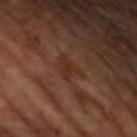Impression: The lesion was photographed on a routine skin check and not biopsied; there is no pathology result. Clinical summary: The patient is a male in their mid- to late 50s. The total-body-photography lesion software estimated an area of roughly 6 mm², an outline eccentricity of about 0.7 (0 = round, 1 = elongated), and a shape-asymmetry score of about 0.45 (0 = symmetric). And it measured a lesion color around L≈30 a*≈19 b*≈26 in CIELAB, roughly 5 lightness units darker than nearby skin, and a normalized border contrast of about 5.5. The analysis additionally found a border-irregularity index near 5/10, internal color variation of about 1 on a 0–10 scale, and a peripheral color-asymmetry measure near 0.5. It also reported a classifier nevus-likeness of about 0/100 and lesion-presence confidence of about 95/100. A 15 mm crop from a total-body photograph taken for skin-cancer surveillance. Imaged with cross-polarized lighting. The lesion is located on the arm. The recorded lesion diameter is about 3.5 mm.